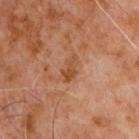A lesion tile, about 15 mm wide, cut from a 3D total-body photograph.
The lesion is on the chest.
A male patient, aged 58–62.